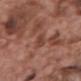Q: Is there a histopathology result?
A: no biopsy performed (imaged during a skin exam)
Q: What kind of image is this?
A: 15 mm crop, total-body photography
Q: What did automated image analysis measure?
A: roughly 8 lightness units darker than nearby skin and a normalized lesion–skin contrast near 6.5; a nevus-likeness score of about 0/100 and lesion-presence confidence of about 85/100
Q: What is the lesion's diameter?
A: about 3 mm
Q: Lesion location?
A: the upper back
Q: What are the patient's age and sex?
A: male, about 70 years old